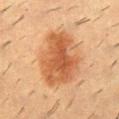notes: no biopsy performed (imaged during a skin exam)
subject: male, aged around 55
illumination: cross-polarized illumination
anatomic site: the mid back
automated lesion analysis: a footprint of about 28 mm² and two-axis asymmetry of about 0.2; a color-variation rating of about 5/10 and radial color variation of about 1.5
image source: 15 mm crop, total-body photography